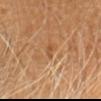Clinical impression: Recorded during total-body skin imaging; not selected for excision or biopsy. Acquisition and patient details: The lesion is located on the head or neck. A region of skin cropped from a whole-body photographic capture, roughly 15 mm wide. A male patient, aged 58–62.